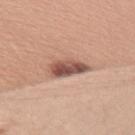Q: Was this lesion biopsied?
A: no biopsy performed (imaged during a skin exam)
Q: Who is the patient?
A: female, aged approximately 25
Q: Where on the body is the lesion?
A: the arm
Q: What is the imaging modality?
A: 15 mm crop, total-body photography